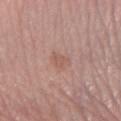The lesion was tiled from a total-body skin photograph and was not biopsied.
From the leg.
A female patient in their mid-60s.
The lesion's longest dimension is about 3 mm.
This image is a 15 mm lesion crop taken from a total-body photograph.
The tile uses white-light illumination.